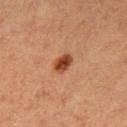notes — total-body-photography surveillance lesion; no biopsy
location — the right thigh
automated lesion analysis — border irregularity of about 1.5 on a 0–10 scale, a within-lesion color-variation index near 3/10, and radial color variation of about 1
image source — total-body-photography crop, ~15 mm field of view
patient — female, about 40 years old
tile lighting — cross-polarized illumination